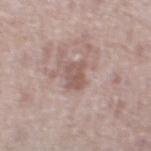Captured during whole-body skin photography for melanoma surveillance; the lesion was not biopsied.
The lesion is on the right thigh.
Longest diameter approximately 3 mm.
This is a white-light tile.
A female subject, aged 58–62.
Cropped from a whole-body photographic skin survey; the tile spans about 15 mm.